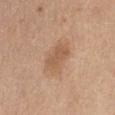follow-up: total-body-photography surveillance lesion; no biopsy
subject: female, in their mid-40s
size: about 4 mm
illumination: white-light illumination
automated metrics: a mean CIELAB color near L≈57 a*≈19 b*≈33, roughly 8 lightness units darker than nearby skin, and a lesion-to-skin contrast of about 6 (normalized; higher = more distinct); border irregularity of about 1.5 on a 0–10 scale, internal color variation of about 2 on a 0–10 scale, and a peripheral color-asymmetry measure near 0.5
imaging modality: ~15 mm tile from a whole-body skin photo
anatomic site: the chest